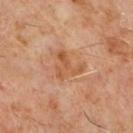Q: Was a biopsy performed?
A: total-body-photography surveillance lesion; no biopsy
Q: What are the patient's age and sex?
A: male, aged 58–62
Q: What is the anatomic site?
A: the chest
Q: Automated lesion metrics?
A: a lesion color around L≈51 a*≈22 b*≈34 in CIELAB, a lesion–skin lightness drop of about 7, and a normalized lesion–skin contrast near 6; a border-irregularity index near 8/10
Q: How large is the lesion?
A: about 4 mm
Q: How was this image acquired?
A: ~15 mm tile from a whole-body skin photo